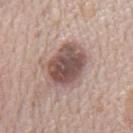workup: no biopsy performed (imaged during a skin exam)
body site: the mid back
image: total-body-photography crop, ~15 mm field of view
patient: male, aged around 70